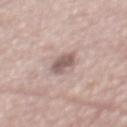| field | value |
|---|---|
| notes | no biopsy performed (imaged during a skin exam) |
| image source | ~15 mm tile from a whole-body skin photo |
| body site | the mid back |
| tile lighting | white-light |
| size | about 4 mm |
| subject | male, about 75 years old |